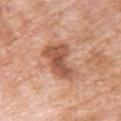Captured during whole-body skin photography for melanoma surveillance; the lesion was not biopsied. The lesion-visualizer software estimated a lesion area of about 14 mm², an eccentricity of roughly 0.75, and a shape-asymmetry score of about 0.4 (0 = symmetric). The lesion is on the back. A female subject in their mid-70s. A region of skin cropped from a whole-body photographic capture, roughly 15 mm wide. This is a white-light tile.